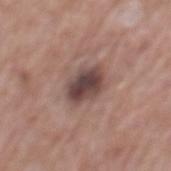Clinical impression: Captured during whole-body skin photography for melanoma surveillance; the lesion was not biopsied. Image and clinical context: The total-body-photography lesion software estimated lesion-presence confidence of about 100/100. The subject is a male in their mid-70s. A 15 mm close-up tile from a total-body photography series done for melanoma screening. About 4 mm across. On the mid back.